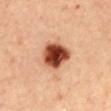This is a cross-polarized tile. Automated image analysis of the tile measured a footprint of about 12 mm² and a shape eccentricity near 0.7. It also reported border irregularity of about 1.5 on a 0–10 scale, a within-lesion color-variation index near 7.5/10, and radial color variation of about 2. The analysis additionally found a nevus-likeness score of about 100/100 and a detector confidence of about 100 out of 100 that the crop contains a lesion. The lesion is located on the mid back. The patient is a female aged 43–47. Cropped from a whole-body photographic skin survey; the tile spans about 15 mm. Measured at roughly 4.5 mm in maximum diameter.